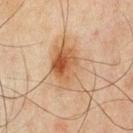Automated image analysis of the tile measured an area of roughly 18 mm², a shape eccentricity near 0.8, and a symmetry-axis asymmetry near 0.25. It also reported an average lesion color of about L≈47 a*≈17 b*≈30 (CIELAB) and a lesion-to-skin contrast of about 8 (normalized; higher = more distinct). The analysis additionally found border irregularity of about 4 on a 0–10 scale, a within-lesion color-variation index near 9/10, and peripheral color asymmetry of about 3. It also reported lesion-presence confidence of about 100/100.
A close-up tile cropped from a whole-body skin photograph, about 15 mm across.
The lesion is located on the front of the torso.
A male patient in their mid-40s.
Measured at roughly 6.5 mm in maximum diameter.
Imaged with cross-polarized lighting.